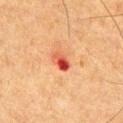Findings:
- follow-up — no biopsy performed (imaged during a skin exam)
- subject — male, in their mid-60s
- image — ~15 mm tile from a whole-body skin photo
- location — the front of the torso
- diameter — ≈2.5 mm